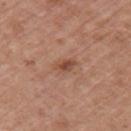The lesion was tiled from a total-body skin photograph and was not biopsied.
An algorithmic analysis of the crop reported an average lesion color of about L≈48 a*≈22 b*≈30 (CIELAB), a lesion–skin lightness drop of about 10, and a normalized lesion–skin contrast near 7.5.
A female patient, aged 38 to 42.
A 15 mm crop from a total-body photograph taken for skin-cancer surveillance.
Imaged with white-light lighting.
On the left upper arm.